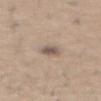Imaged during a routine full-body skin examination; the lesion was not biopsied and no histopathology is available. About 2.5 mm across. A close-up tile cropped from a whole-body skin photograph, about 15 mm across. A male patient, aged around 60. The lesion is located on the abdomen.